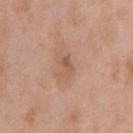Q: Was this lesion biopsied?
A: catalogued during a skin exam; not biopsied
Q: What is the imaging modality?
A: total-body-photography crop, ~15 mm field of view
Q: Who is the patient?
A: male, approximately 65 years of age
Q: How large is the lesion?
A: ≈2.5 mm
Q: Lesion location?
A: the chest
Q: What lighting was used for the tile?
A: white-light